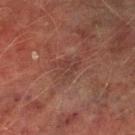The lesion was photographed on a routine skin check and not biopsied; there is no pathology result. A lesion tile, about 15 mm wide, cut from a 3D total-body photograph. Captured under cross-polarized illumination. A male patient, aged around 75. About 3.5 mm across. The total-body-photography lesion software estimated a lesion–skin lightness drop of about 5 and a normalized border contrast of about 5. The analysis additionally found border irregularity of about 5 on a 0–10 scale and a color-variation rating of about 2/10. And it measured an automated nevus-likeness rating near 0 out of 100 and lesion-presence confidence of about 95/100. From the leg.